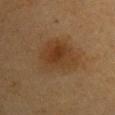Q: Is there a histopathology result?
A: imaged on a skin check; not biopsied
Q: What lighting was used for the tile?
A: cross-polarized illumination
Q: Lesion size?
A: ≈6 mm
Q: Where on the body is the lesion?
A: the right upper arm
Q: What did automated image analysis measure?
A: an automated nevus-likeness rating near 90 out of 100
Q: Who is the patient?
A: female, in their mid-50s
Q: What is the imaging modality?
A: ~15 mm crop, total-body skin-cancer survey